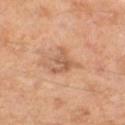{
  "biopsy_status": "not biopsied; imaged during a skin examination",
  "lighting": "cross-polarized",
  "site": "right thigh",
  "image": {
    "source": "total-body photography crop",
    "field_of_view_mm": 15
  },
  "lesion_size": {
    "long_diameter_mm_approx": 3.5
  },
  "patient": {
    "sex": "male",
    "age_approx": 55
  }
}